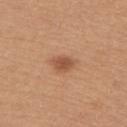Notes:
* follow-up · no biopsy performed (imaged during a skin exam)
* lesion size · ~2.5 mm (longest diameter)
* body site · the upper back
* image · ~15 mm crop, total-body skin-cancer survey
* TBP lesion metrics · a lesion area of about 4.5 mm², a shape eccentricity near 0.7, and a symmetry-axis asymmetry near 0.25; a classifier nevus-likeness of about 90/100 and a lesion-detection confidence of about 100/100
* subject · female, about 45 years old
* illumination · white-light illumination